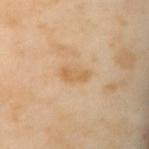follow-up = catalogued during a skin exam; not biopsied
diameter = ~3.5 mm (longest diameter)
lighting = cross-polarized
subject = female, approximately 55 years of age
image = 15 mm crop, total-body photography
location = the mid back
automated lesion analysis = a lesion area of about 4.5 mm² and an eccentricity of roughly 0.9; a lesion color around L≈64 a*≈18 b*≈40 in CIELAB, about 7 CIELAB-L* units darker than the surrounding skin, and a normalized lesion–skin contrast near 6; a border-irregularity rating of about 3/10, a within-lesion color-variation index near 2.5/10, and radial color variation of about 1